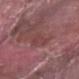| field | value |
|---|---|
| workup | total-body-photography surveillance lesion; no biopsy |
| lesion size | about 4 mm |
| image | total-body-photography crop, ~15 mm field of view |
| patient | male, aged approximately 40 |
| lighting | white-light |
| image-analysis metrics | a lesion color around L≈42 a*≈25 b*≈20 in CIELAB, about 6 CIELAB-L* units darker than the surrounding skin, and a normalized lesion–skin contrast near 5; a border-irregularity index near 5.5/10 and a peripheral color-asymmetry measure near 0; a detector confidence of about 60 out of 100 that the crop contains a lesion |
| location | the right forearm |